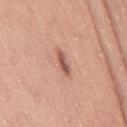No biopsy was performed on this lesion — it was imaged during a full skin examination and was not determined to be concerning.
From the right thigh.
A 15 mm close-up tile from a total-body photography series done for melanoma screening.
This is a white-light tile.
Measured at roughly 3.5 mm in maximum diameter.
The subject is a female about 45 years old.